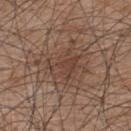biopsy status = no biopsy performed (imaged during a skin exam) | size = ≈6.5 mm | illumination = white-light | subject = male, aged around 45 | location = the upper back | imaging modality = ~15 mm tile from a whole-body skin photo.